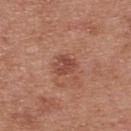Imaged during a routine full-body skin examination; the lesion was not biopsied and no histopathology is available.
The subject is a male approximately 30 years of age.
Located on the upper back.
Automated image analysis of the tile measured an eccentricity of roughly 0.5 and a shape-asymmetry score of about 0.3 (0 = symmetric). And it measured an average lesion color of about L≈47 a*≈25 b*≈28 (CIELAB), a lesion–skin lightness drop of about 9, and a normalized lesion–skin contrast near 7. And it measured a nevus-likeness score of about 15/100 and a detector confidence of about 100 out of 100 that the crop contains a lesion.
A close-up tile cropped from a whole-body skin photograph, about 15 mm across.
Approximately 2.5 mm at its widest.
Captured under white-light illumination.